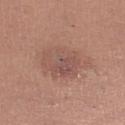Clinical impression:
Captured during whole-body skin photography for melanoma surveillance; the lesion was not biopsied.